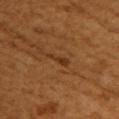Findings:
* follow-up — imaged on a skin check; not biopsied
* lesion diameter — about 3 mm
* TBP lesion metrics — a mean CIELAB color near L≈35 a*≈23 b*≈36 and roughly 7 lightness units darker than nearby skin; internal color variation of about 0 on a 0–10 scale and peripheral color asymmetry of about 0
* patient — female, in their mid- to late 50s
* site — the right upper arm
* image — ~15 mm tile from a whole-body skin photo
* illumination — cross-polarized illumination